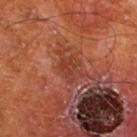The lesion was photographed on a routine skin check and not biopsied; there is no pathology result.
Cropped from a whole-body photographic skin survey; the tile spans about 15 mm.
The lesion's longest dimension is about 3 mm.
On the left lower leg.
The patient is a male in their mid-60s.